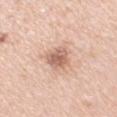Assessment: The lesion was photographed on a routine skin check and not biopsied; there is no pathology result. Image and clinical context: A 15 mm close-up tile from a total-body photography series done for melanoma screening. The tile uses white-light illumination. The lesion is on the arm. A female patient in their 40s. The total-body-photography lesion software estimated an area of roughly 7 mm² and an eccentricity of roughly 0.7. The software also gave a lesion color around L≈63 a*≈21 b*≈28 in CIELAB, a lesion–skin lightness drop of about 13, and a normalized lesion–skin contrast near 7.5. The analysis additionally found a border-irregularity rating of about 2.5/10, internal color variation of about 4 on a 0–10 scale, and a peripheral color-asymmetry measure near 1.5. It also reported a classifier nevus-likeness of about 40/100.